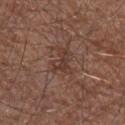workup: no biopsy performed (imaged during a skin exam)
patient: male, in their mid- to late 60s
lighting: white-light
size: ~3 mm (longest diameter)
imaging modality: total-body-photography crop, ~15 mm field of view
location: the left forearm
automated metrics: lesion-presence confidence of about 95/100